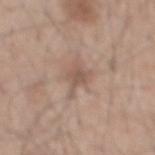Assessment:
Captured during whole-body skin photography for melanoma surveillance; the lesion was not biopsied.
Acquisition and patient details:
The tile uses white-light illumination. A lesion tile, about 15 mm wide, cut from a 3D total-body photograph. A male patient in their mid-40s. Measured at roughly 4 mm in maximum diameter. The lesion is on the mid back.